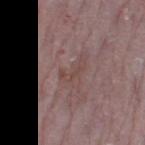A 15 mm crop from a total-body photograph taken for skin-cancer surveillance.
A female subject approximately 65 years of age.
On the left thigh.